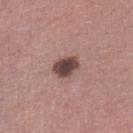Assessment: The lesion was photographed on a routine skin check and not biopsied; there is no pathology result. Context: The lesion-visualizer software estimated a footprint of about 6 mm², an eccentricity of roughly 0.65, and a shape-asymmetry score of about 0.25 (0 = symmetric). A female subject, aged 58 to 62. A 15 mm crop from a total-body photograph taken for skin-cancer surveillance. On the left lower leg. Imaged with white-light lighting. The lesion's longest dimension is about 3 mm.